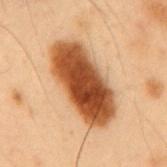Part of a total-body skin-imaging series; this lesion was reviewed on a skin check and was not flagged for biopsy. The subject is a male in their mid-50s. Automated tile analysis of the lesion measured a lesion area of about 30 mm², an outline eccentricity of about 0.9 (0 = round, 1 = elongated), and a shape-asymmetry score of about 0.1 (0 = symmetric). The software also gave a mean CIELAB color near L≈41 a*≈22 b*≈34, roughly 19 lightness units darker than nearby skin, and a lesion-to-skin contrast of about 14 (normalized; higher = more distinct). And it measured internal color variation of about 6.5 on a 0–10 scale and a peripheral color-asymmetry measure near 2. The analysis additionally found a detector confidence of about 100 out of 100 that the crop contains a lesion. A roughly 15 mm field-of-view crop from a total-body skin photograph. The lesion is located on the mid back. Captured under cross-polarized illumination.